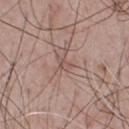workup — total-body-photography surveillance lesion; no biopsy
size — ≈2 mm
automated metrics — a footprint of about 2 mm², an eccentricity of roughly 0.75, and a shape-asymmetry score of about 0.4 (0 = symmetric); an average lesion color of about L≈52 a*≈19 b*≈23 (CIELAB), roughly 7 lightness units darker than nearby skin, and a normalized border contrast of about 5; an automated nevus-likeness rating near 0 out of 100 and lesion-presence confidence of about 55/100
tile lighting — white-light illumination
patient — male, aged 43–47
acquisition — ~15 mm tile from a whole-body skin photo
anatomic site — the upper back